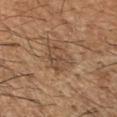Clinical impression: The lesion was tiled from a total-body skin photograph and was not biopsied. Background: A 15 mm close-up extracted from a 3D total-body photography capture. The lesion is located on the right forearm. Captured under cross-polarized illumination. A male subject, roughly 65 years of age.